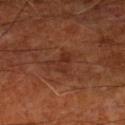Assessment: The lesion was photographed on a routine skin check and not biopsied; there is no pathology result. Image and clinical context: A male subject, aged approximately 65. The lesion is on the left lower leg. This image is a 15 mm lesion crop taken from a total-body photograph.